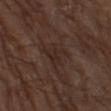  automated_metrics:
    cielab_L: 25
    cielab_a: 15
    cielab_b: 21
    vs_skin_darker_L: 5.0
    vs_skin_contrast_norm: 5.5
  lighting: white-light
  lesion_size:
    long_diameter_mm_approx: 3.0
  patient:
    sex: male
    age_approx: 75
  site: left thigh
  image:
    source: total-body photography crop
    field_of_view_mm: 15